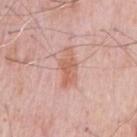notes = catalogued during a skin exam; not biopsied | site = the chest | lighting = white-light illumination | patient = male, about 80 years old | image-analysis metrics = a lesion color around L≈62 a*≈24 b*≈29 in CIELAB and about 9 CIELAB-L* units darker than the surrounding skin; a peripheral color-asymmetry measure near 1; a nevus-likeness score of about 10/100 and a detector confidence of about 100 out of 100 that the crop contains a lesion | image source = 15 mm crop, total-body photography.